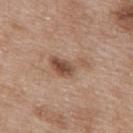Captured during whole-body skin photography for melanoma surveillance; the lesion was not biopsied. The lesion is on the upper back. A female patient aged 38–42. Approximately 5 mm at its widest. This is a white-light tile. Automated tile analysis of the lesion measured an area of roughly 7 mm², an eccentricity of roughly 0.85, and a shape-asymmetry score of about 0.55 (0 = symmetric). The analysis additionally found an average lesion color of about L≈50 a*≈19 b*≈28 (CIELAB), a lesion–skin lightness drop of about 11, and a normalized border contrast of about 8. The analysis additionally found a border-irregularity index near 6/10 and peripheral color asymmetry of about 1.5. And it measured lesion-presence confidence of about 100/100. A 15 mm close-up tile from a total-body photography series done for melanoma screening.